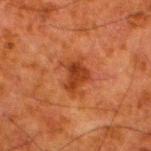Part of a total-body skin-imaging series; this lesion was reviewed on a skin check and was not flagged for biopsy.
The lesion is located on the left lower leg.
Imaged with cross-polarized lighting.
A region of skin cropped from a whole-body photographic capture, roughly 15 mm wide.
The patient is a male aged 78 to 82.
The recorded lesion diameter is about 3.5 mm.